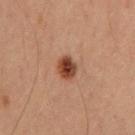Part of a total-body skin-imaging series; this lesion was reviewed on a skin check and was not flagged for biopsy.
This is a cross-polarized tile.
A male patient approximately 60 years of age.
Cropped from a total-body skin-imaging series; the visible field is about 15 mm.
Automated image analysis of the tile measured a border-irregularity rating of about 1.5/10, a color-variation rating of about 5.5/10, and peripheral color asymmetry of about 2. It also reported an automated nevus-likeness rating near 100 out of 100 and lesion-presence confidence of about 100/100.
Longest diameter approximately 3 mm.
On the chest.